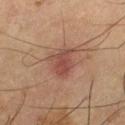biopsy_status: not biopsied; imaged during a skin examination
patient:
  sex: male
  age_approx: 65
site: left thigh
image:
  source: total-body photography crop
  field_of_view_mm: 15
lighting: cross-polarized
automated_metrics:
  cielab_L: 37
  cielab_a: 19
  cielab_b: 22
  vs_skin_contrast_norm: 7.0
  border_irregularity_0_10: 3.0
  color_variation_0_10: 3.5
  peripheral_color_asymmetry: 1.0
  lesion_detection_confidence_0_100: 100